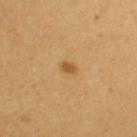This lesion was catalogued during total-body skin photography and was not selected for biopsy.
The patient is a female in their mid-60s.
The lesion is located on the right upper arm.
A 15 mm close-up tile from a total-body photography series done for melanoma screening.